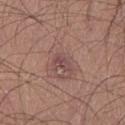follow-up: total-body-photography surveillance lesion; no biopsy
image source: ~15 mm tile from a whole-body skin photo
TBP lesion metrics: a lesion area of about 3.5 mm², an outline eccentricity of about 0.85 (0 = round, 1 = elongated), and a symmetry-axis asymmetry near 0.3; a mean CIELAB color near L≈46 a*≈21 b*≈19, roughly 8 lightness units darker than nearby skin, and a normalized lesion–skin contrast near 6.5; a border-irregularity index near 3/10, a color-variation rating of about 3/10, and a peripheral color-asymmetry measure near 1
subject: male, aged 23–27
site: the leg
lighting: white-light illumination